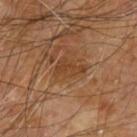Case summary:
- follow-up — imaged on a skin check; not biopsied
- anatomic site — the arm
- acquisition — 15 mm crop, total-body photography
- patient — male, aged approximately 65